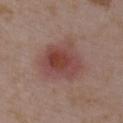The lesion was photographed on a routine skin check and not biopsied; there is no pathology result. Captured under white-light illumination. The lesion-visualizer software estimated an automated nevus-likeness rating near 95 out of 100. A 15 mm crop from a total-body photograph taken for skin-cancer surveillance. The subject is a female aged 33–37. Located on the chest.